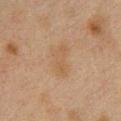Case summary:
– lighting — cross-polarized
– site — the front of the torso
– size — ~4.5 mm (longest diameter)
– image source — ~15 mm crop, total-body skin-cancer survey
– subject — female, about 40 years old